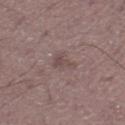Background: On the left thigh. The tile uses white-light illumination. A lesion tile, about 15 mm wide, cut from a 3D total-body photograph. A male subject about 60 years old.